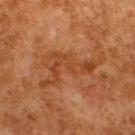– follow-up — total-body-photography surveillance lesion; no biopsy
– anatomic site — the upper back
– TBP lesion metrics — an eccentricity of roughly 0.9; a lesion color around L≈34 a*≈21 b*≈31 in CIELAB, roughly 6 lightness units darker than nearby skin, and a normalized border contrast of about 6; a border-irregularity index near 10/10, a within-lesion color-variation index near 3.5/10, and peripheral color asymmetry of about 1
– image source — total-body-photography crop, ~15 mm field of view
– size — ~8.5 mm (longest diameter)
– subject — male, aged around 80
– tile lighting — cross-polarized